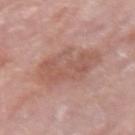follow-up: imaged on a skin check; not biopsied
image-analysis metrics: a shape eccentricity near 0.8 and a shape-asymmetry score of about 0.25 (0 = symmetric); a lesion color around L≈56 a*≈22 b*≈26 in CIELAB and roughly 8 lightness units darker than nearby skin; a classifier nevus-likeness of about 0/100 and a detector confidence of about 100 out of 100 that the crop contains a lesion
lesion diameter: about 7 mm
subject: female, aged 63–67
anatomic site: the right forearm
tile lighting: white-light illumination
acquisition: ~15 mm crop, total-body skin-cancer survey